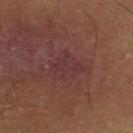Acquisition and patient details: The lesion is located on the left lower leg. A male subject, aged 58–62. The tile uses cross-polarized illumination. Measured at roughly 3 mm in maximum diameter. This image is a 15 mm lesion crop taken from a total-body photograph.